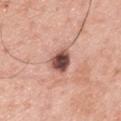This lesion was catalogued during total-body skin photography and was not selected for biopsy. The lesion is on the mid back. A region of skin cropped from a whole-body photographic capture, roughly 15 mm wide. Captured under white-light illumination. The patient is a male aged around 60.